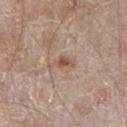Q: Was this lesion biopsied?
A: catalogued during a skin exam; not biopsied
Q: Automated lesion metrics?
A: an area of roughly 4.5 mm² and two-axis asymmetry of about 0.35
Q: What kind of image is this?
A: total-body-photography crop, ~15 mm field of view
Q: What is the lesion's diameter?
A: about 3.5 mm
Q: What lighting was used for the tile?
A: white-light
Q: Where on the body is the lesion?
A: the chest
Q: What are the patient's age and sex?
A: male, in their mid- to late 60s